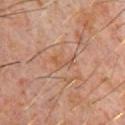biopsy status = total-body-photography surveillance lesion; no biopsy | body site = the chest | acquisition = ~15 mm tile from a whole-body skin photo | subject = male, in their 60s.